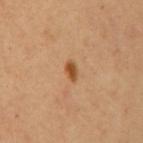Q: Was this lesion biopsied?
A: imaged on a skin check; not biopsied
Q: Lesion size?
A: ~2.5 mm (longest diameter)
Q: How was this image acquired?
A: ~15 mm crop, total-body skin-cancer survey
Q: What are the patient's age and sex?
A: female
Q: Lesion location?
A: the mid back
Q: Illumination type?
A: cross-polarized